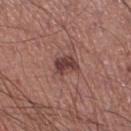biopsy status — no biopsy performed (imaged during a skin exam)
automated lesion analysis — border irregularity of about 2.5 on a 0–10 scale and a color-variation rating of about 3.5/10
acquisition — ~15 mm crop, total-body skin-cancer survey
location — the leg
patient — male, in their mid-40s